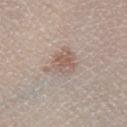The lesion is on the right lower leg. This is a white-light tile. The lesion's longest dimension is about 4 mm. A male subject about 35 years old. A lesion tile, about 15 mm wide, cut from a 3D total-body photograph.